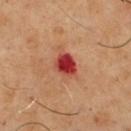Case summary:
– follow-up: total-body-photography surveillance lesion; no biopsy
– site: the chest
– imaging modality: ~15 mm crop, total-body skin-cancer survey
– subject: male, aged approximately 50
– illumination: cross-polarized
– automated metrics: a footprint of about 5.5 mm², an outline eccentricity of about 0.6 (0 = round, 1 = elongated), and two-axis asymmetry of about 0.2; an average lesion color of about L≈42 a*≈39 b*≈32 (CIELAB), roughly 17 lightness units darker than nearby skin, and a normalized lesion–skin contrast near 12.5; border irregularity of about 1.5 on a 0–10 scale, a within-lesion color-variation index near 4.5/10, and radial color variation of about 1.5